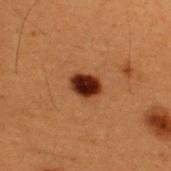Q: Automated lesion metrics?
A: an area of roughly 6 mm², an outline eccentricity of about 0.7 (0 = round, 1 = elongated), and two-axis asymmetry of about 0.2; a lesion color around L≈24 a*≈22 b*≈26 in CIELAB, roughly 17 lightness units darker than nearby skin, and a normalized lesion–skin contrast near 17; a border-irregularity rating of about 1.5/10, a color-variation rating of about 4.5/10, and a peripheral color-asymmetry measure near 1
Q: Who is the patient?
A: male, aged around 50
Q: What is the anatomic site?
A: the upper back
Q: Illumination type?
A: cross-polarized illumination
Q: Lesion size?
A: about 3 mm
Q: What is the imaging modality?
A: ~15 mm tile from a whole-body skin photo